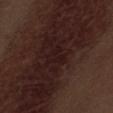Imaged during a routine full-body skin examination; the lesion was not biopsied and no histopathology is available. From the abdomen. About 3 mm across. Captured under white-light illumination. Cropped from a total-body skin-imaging series; the visible field is about 15 mm. The subject is a male aged approximately 70.